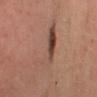{"biopsy_status": "not biopsied; imaged during a skin examination", "site": "chest", "lighting": "cross-polarized", "lesion_size": {"long_diameter_mm_approx": 6.5}, "patient": {"sex": "female", "age_approx": 40}, "image": {"source": "total-body photography crop", "field_of_view_mm": 15}}